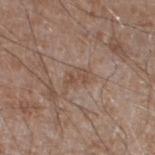follow-up: catalogued during a skin exam; not biopsied | imaging modality: total-body-photography crop, ~15 mm field of view | lighting: white-light illumination | subject: male, about 60 years old | anatomic site: the left lower leg | image-analysis metrics: a footprint of about 2.5 mm² and a shape eccentricity near 0.85; about 7 CIELAB-L* units darker than the surrounding skin; border irregularity of about 6 on a 0–10 scale and a color-variation rating of about 0/10; a nevus-likeness score of about 0/100 and a lesion-detection confidence of about 100/100.